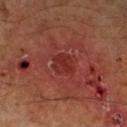Q: What is the imaging modality?
A: ~15 mm tile from a whole-body skin photo
Q: Where on the body is the lesion?
A: the left lower leg
Q: How large is the lesion?
A: ~2.5 mm (longest diameter)
Q: How was the tile lit?
A: cross-polarized
Q: Who is the patient?
A: male, approximately 60 years of age
Q: Automated lesion metrics?
A: a lesion area of about 5.5 mm², an eccentricity of roughly 0.5, and a symmetry-axis asymmetry near 0.2